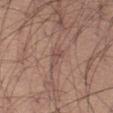workup — no biopsy performed (imaged during a skin exam)
site — the left thigh
patient — male, aged around 45
lesion diameter — ≈3 mm
acquisition — ~15 mm tile from a whole-body skin photo
TBP lesion metrics — a symmetry-axis asymmetry near 0.5; a lesion color around L≈49 a*≈19 b*≈23 in CIELAB, a lesion–skin lightness drop of about 7, and a lesion-to-skin contrast of about 5.5 (normalized; higher = more distinct); an automated nevus-likeness rating near 0 out of 100 and a lesion-detection confidence of about 80/100
tile lighting — white-light illumination